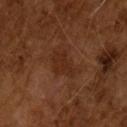The lesion was photographed on a routine skin check and not biopsied; there is no pathology result.
A 15 mm crop from a total-body photograph taken for skin-cancer surveillance.
A male subject, aged approximately 65.
Automated image analysis of the tile measured a nevus-likeness score of about 0/100 and lesion-presence confidence of about 100/100.